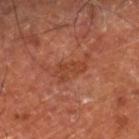The lesion was photographed on a routine skin check and not biopsied; there is no pathology result. Approximately 3.5 mm at its widest. Imaged with cross-polarized lighting. A subject in their mid-60s. The lesion is on the right lower leg. Cropped from a whole-body photographic skin survey; the tile spans about 15 mm.